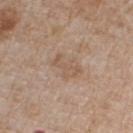Impression: Part of a total-body skin-imaging series; this lesion was reviewed on a skin check and was not flagged for biopsy. Background: This is a white-light tile. An algorithmic analysis of the crop reported a lesion color around L≈56 a*≈15 b*≈29 in CIELAB, roughly 6 lightness units darker than nearby skin, and a normalized border contrast of about 5. The software also gave a lesion-detection confidence of about 100/100. The lesion is located on the chest. A male subject about 65 years old. Cropped from a whole-body photographic skin survey; the tile spans about 15 mm.